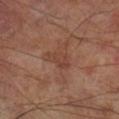A roughly 15 mm field-of-view crop from a total-body skin photograph.
The lesion is on the right lower leg.
Measured at roughly 3.5 mm in maximum diameter.
The tile uses cross-polarized illumination.
A male patient, aged approximately 70.